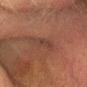workup — total-body-photography surveillance lesion; no biopsy | acquisition — ~15 mm crop, total-body skin-cancer survey | size — ~3.5 mm (longest diameter) | subject — male, about 60 years old | body site — the head or neck.